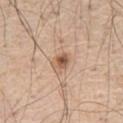biopsy_status: not biopsied; imaged during a skin examination
lesion_size:
  long_diameter_mm_approx: 2.5
site: left thigh
lighting: white-light
automated_metrics:
  border_irregularity_0_10: 2.0
  color_variation_0_10: 5.0
  peripheral_color_asymmetry: 1.5
  nevus_likeness_0_100: 85
  lesion_detection_confidence_0_100: 100
image:
  source: total-body photography crop
  field_of_view_mm: 15
patient:
  sex: male
  age_approx: 70